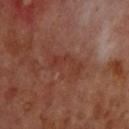| field | value |
|---|---|
| notes | catalogued during a skin exam; not biopsied |
| acquisition | ~15 mm crop, total-body skin-cancer survey |
| subject | male, aged 68 to 72 |
| diameter | ~4.5 mm (longest diameter) |
| site | the back |
| illumination | cross-polarized |
| automated lesion analysis | a mean CIELAB color near L≈36 a*≈24 b*≈27 and about 5 CIELAB-L* units darker than the surrounding skin |